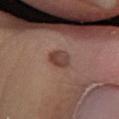Assessment: Imaged during a routine full-body skin examination; the lesion was not biopsied and no histopathology is available. Context: The tile uses white-light illumination. A male subject, aged around 35. Automated image analysis of the tile measured border irregularity of about 1.5 on a 0–10 scale, a within-lesion color-variation index near 3.5/10, and peripheral color asymmetry of about 1. And it measured an automated nevus-likeness rating near 15 out of 100. Longest diameter approximately 3 mm. A roughly 15 mm field-of-view crop from a total-body skin photograph. On the leg.